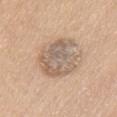Impression: The lesion was tiled from a total-body skin photograph and was not biopsied. Image and clinical context: A female subject, aged around 70. Cropped from a total-body skin-imaging series; the visible field is about 15 mm. The tile uses white-light illumination. From the chest. About 5.5 mm across.